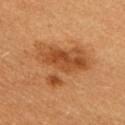| feature | finding |
|---|---|
| biopsy status | imaged on a skin check; not biopsied |
| automated lesion analysis | an area of roughly 22 mm², an outline eccentricity of about 0.6 (0 = round, 1 = elongated), and a symmetry-axis asymmetry near 0.55; an automated nevus-likeness rating near 45 out of 100 |
| lighting | cross-polarized illumination |
| diameter | about 6.5 mm |
| patient | female, roughly 50 years of age |
| image source | 15 mm crop, total-body photography |